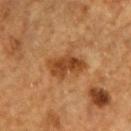follow-up = imaged on a skin check; not biopsied
lesion size = about 4.5 mm
lighting = cross-polarized illumination
image source = ~15 mm crop, total-body skin-cancer survey
subject = male, roughly 85 years of age or older
anatomic site = the head or neck
automated lesion analysis = an eccentricity of roughly 0.8 and two-axis asymmetry of about 0.2; border irregularity of about 3 on a 0–10 scale; a classifier nevus-likeness of about 60/100 and lesion-presence confidence of about 100/100